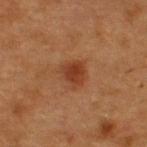notes: no biopsy performed (imaged during a skin exam)
patient: female, approximately 40 years of age
TBP lesion metrics: a nevus-likeness score of about 95/100 and lesion-presence confidence of about 100/100
acquisition: total-body-photography crop, ~15 mm field of view
anatomic site: the upper back
lesion size: about 3 mm
tile lighting: cross-polarized illumination